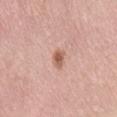Imaged during a routine full-body skin examination; the lesion was not biopsied and no histopathology is available. The lesion is on the back. Measured at roughly 2.5 mm in maximum diameter. The patient is a female about 40 years old. A region of skin cropped from a whole-body photographic capture, roughly 15 mm wide. Imaged with white-light lighting.